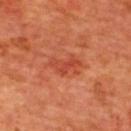{
  "biopsy_status": "not biopsied; imaged during a skin examination"
}